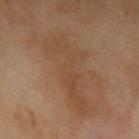Recorded during total-body skin imaging; not selected for excision or biopsy. Imaged with cross-polarized lighting. A roughly 15 mm field-of-view crop from a total-body skin photograph. The total-body-photography lesion software estimated a mean CIELAB color near L≈44 a*≈18 b*≈30, a lesion–skin lightness drop of about 5, and a normalized border contrast of about 5. The analysis additionally found border irregularity of about 8.5 on a 0–10 scale and peripheral color asymmetry of about 1. It also reported lesion-presence confidence of about 100/100. A male subject, aged 68–72. Longest diameter approximately 8.5 mm. The lesion is located on the left upper arm.